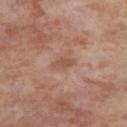Located on the left thigh.
A region of skin cropped from a whole-body photographic capture, roughly 15 mm wide.
A female subject, roughly 55 years of age.